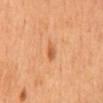Q: Is there a histopathology result?
A: no biopsy performed (imaged during a skin exam)
Q: What lighting was used for the tile?
A: cross-polarized
Q: What are the patient's age and sex?
A: female
Q: What is the imaging modality?
A: 15 mm crop, total-body photography
Q: Lesion location?
A: the mid back
Q: What is the lesion's diameter?
A: ≈2.5 mm